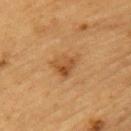Imaged during a routine full-body skin examination; the lesion was not biopsied and no histopathology is available. Measured at roughly 3 mm in maximum diameter. A close-up tile cropped from a whole-body skin photograph, about 15 mm across. Automated image analysis of the tile measured a lesion area of about 6 mm² and an eccentricity of roughly 0.65. The software also gave border irregularity of about 4 on a 0–10 scale, a color-variation rating of about 6/10, and a peripheral color-asymmetry measure near 2. And it measured a classifier nevus-likeness of about 35/100 and lesion-presence confidence of about 100/100. On the left upper arm. A male patient approximately 85 years of age or older. Imaged with cross-polarized lighting.